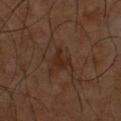Assessment:
No biopsy was performed on this lesion — it was imaged during a full skin examination and was not determined to be concerning.
Background:
A male patient roughly 60 years of age. An algorithmic analysis of the crop reported a lesion color around L≈23 a*≈16 b*≈24 in CIELAB and a lesion-to-skin contrast of about 7 (normalized; higher = more distinct). And it measured a classifier nevus-likeness of about 0/100. A 15 mm crop from a total-body photograph taken for skin-cancer surveillance. This is a cross-polarized tile. The lesion's longest dimension is about 2.5 mm. The lesion is on the chest.